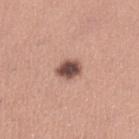  biopsy_status: not biopsied; imaged during a skin examination
  image:
    source: total-body photography crop
    field_of_view_mm: 15
  patient:
    sex: female
    age_approx: 30
  lesion_size:
    long_diameter_mm_approx: 3.0
  lighting: white-light
  site: left lower leg